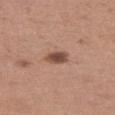* follow-up: imaged on a skin check; not biopsied
* diameter: ~3 mm (longest diameter)
* body site: the left thigh
* illumination: white-light
* automated lesion analysis: an area of roughly 4.5 mm², an eccentricity of roughly 0.75, and a symmetry-axis asymmetry near 0.25; a border-irregularity rating of about 2/10, internal color variation of about 4 on a 0–10 scale, and peripheral color asymmetry of about 1
* subject: female, aged approximately 30
* image source: ~15 mm tile from a whole-body skin photo